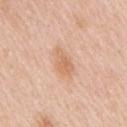Imaged with white-light lighting. From the right upper arm. A 15 mm close-up tile from a total-body photography series done for melanoma screening. A female patient, aged 43 to 47. Measured at roughly 3.5 mm in maximum diameter.